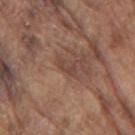Imaged during a routine full-body skin examination; the lesion was not biopsied and no histopathology is available.
Located on the arm.
Captured under white-light illumination.
The patient is a male aged 78 to 82.
The lesion-visualizer software estimated a mean CIELAB color near L≈43 a*≈19 b*≈25 and roughly 7 lightness units darker than nearby skin.
A 15 mm crop from a total-body photograph taken for skin-cancer surveillance.
About 3 mm across.